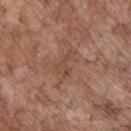Findings:
– follow-up: imaged on a skin check; not biopsied